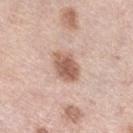Background: From the leg. Cropped from a whole-body photographic skin survey; the tile spans about 15 mm. The lesion's longest dimension is about 4 mm. A female subject, in their mid- to late 60s. Captured under white-light illumination. The total-body-photography lesion software estimated a border-irregularity rating of about 1.5/10, a within-lesion color-variation index near 3.5/10, and peripheral color asymmetry of about 1.5.